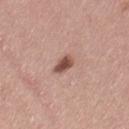Clinical impression:
No biopsy was performed on this lesion — it was imaged during a full skin examination and was not determined to be concerning.
Acquisition and patient details:
A female patient aged 28 to 32. Approximately 2.5 mm at its widest. A lesion tile, about 15 mm wide, cut from a 3D total-body photograph. From the leg. This is a white-light tile.